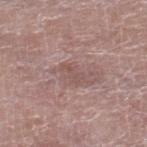No biopsy was performed on this lesion — it was imaged during a full skin examination and was not determined to be concerning.
Located on the leg.
Automated tile analysis of the lesion measured a lesion color around L≈51 a*≈19 b*≈22 in CIELAB, a lesion–skin lightness drop of about 6, and a normalized border contrast of about 5. It also reported a border-irregularity rating of about 6/10, internal color variation of about 0 on a 0–10 scale, and radial color variation of about 0.
A region of skin cropped from a whole-body photographic capture, roughly 15 mm wide.
Longest diameter approximately 2.5 mm.
This is a white-light tile.
The patient is a male about 80 years old.